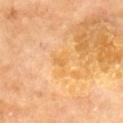| field | value |
|---|---|
| notes | catalogued during a skin exam; not biopsied |
| location | the back |
| patient | male, aged 68–72 |
| acquisition | ~15 mm tile from a whole-body skin photo |